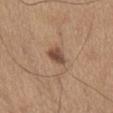location=the abdomen
acquisition=~15 mm tile from a whole-body skin photo
patient=male, aged approximately 65